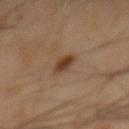Assessment:
Recorded during total-body skin imaging; not selected for excision or biopsy.
Image and clinical context:
The patient is a male in their mid- to late 40s. A 15 mm close-up extracted from a 3D total-body photography capture. The lesion is located on the mid back. Automated tile analysis of the lesion measured border irregularity of about 2 on a 0–10 scale and peripheral color asymmetry of about 1.5. The tile uses cross-polarized illumination.